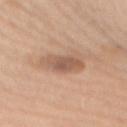Impression: Captured during whole-body skin photography for melanoma surveillance; the lesion was not biopsied. Image and clinical context: Longest diameter approximately 5.5 mm. A roughly 15 mm field-of-view crop from a total-body skin photograph. A female subject aged 68 to 72. The lesion is on the mid back.